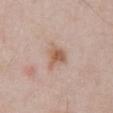Notes:
- notes: imaged on a skin check; not biopsied
- acquisition: total-body-photography crop, ~15 mm field of view
- patient: male, aged 53 to 57
- location: the abdomen
- tile lighting: white-light